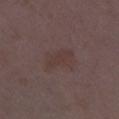notes: imaged on a skin check; not biopsied